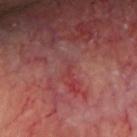Impression: Recorded during total-body skin imaging; not selected for excision or biopsy. Background: Longest diameter approximately 2 mm. The total-body-photography lesion software estimated a lesion area of about 2 mm², an eccentricity of roughly 0.9, and a symmetry-axis asymmetry near 0.45. And it measured a mean CIELAB color near L≈41 a*≈30 b*≈22, about 4 CIELAB-L* units darker than the surrounding skin, and a normalized border contrast of about 3.5. The analysis additionally found a border-irregularity index near 5/10 and radial color variation of about 0. It also reported a classifier nevus-likeness of about 0/100. Located on the head or neck. This is a cross-polarized tile. Cropped from a whole-body photographic skin survey; the tile spans about 15 mm. The subject is a male in their mid- to late 60s.